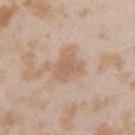This lesion was catalogued during total-body skin photography and was not selected for biopsy.
A 15 mm close-up tile from a total-body photography series done for melanoma screening.
About 3.5 mm across.
From the left upper arm.
Imaged with white-light lighting.
The subject is a female approximately 25 years of age.